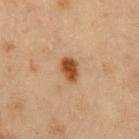{"biopsy_status": "not biopsied; imaged during a skin examination", "image": {"source": "total-body photography crop", "field_of_view_mm": 15}, "site": "front of the torso", "automated_metrics": {"area_mm2_approx": 5.0, "eccentricity": 0.75, "shape_asymmetry": 0.2, "nevus_likeness_0_100": 100, "lesion_detection_confidence_0_100": 100}, "lighting": "cross-polarized", "patient": {"sex": "male", "age_approx": 55}, "lesion_size": {"long_diameter_mm_approx": 3.0}}